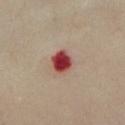patient: female, in their mid-50s
anatomic site: the chest
imaging modality: 15 mm crop, total-body photography